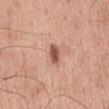Captured during whole-body skin photography for melanoma surveillance; the lesion was not biopsied.
An algorithmic analysis of the crop reported a footprint of about 4 mm², a shape eccentricity near 0.9, and a symmetry-axis asymmetry near 0.2. The analysis additionally found a lesion–skin lightness drop of about 14. The analysis additionally found a classifier nevus-likeness of about 90/100 and a lesion-detection confidence of about 100/100.
A male patient, approximately 60 years of age.
Longest diameter approximately 3.5 mm.
On the back.
A roughly 15 mm field-of-view crop from a total-body skin photograph.
Captured under white-light illumination.